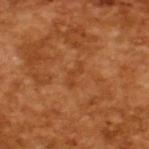The lesion was tiled from a total-body skin photograph and was not biopsied.
Approximately 3 mm at its widest.
Imaged with cross-polarized lighting.
A close-up tile cropped from a whole-body skin photograph, about 15 mm across.
A male subject aged 63–67.